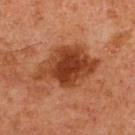Clinical impression: Recorded during total-body skin imaging; not selected for excision or biopsy. Clinical summary: From the chest. This is a cross-polarized tile. The total-body-photography lesion software estimated a footprint of about 25 mm², an eccentricity of roughly 0.75, and two-axis asymmetry of about 0.2. The analysis additionally found a color-variation rating of about 5.5/10 and peripheral color asymmetry of about 1.5. It also reported a nevus-likeness score of about 90/100 and lesion-presence confidence of about 100/100. A male subject, in their 60s. A lesion tile, about 15 mm wide, cut from a 3D total-body photograph.